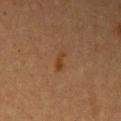The lesion was photographed on a routine skin check and not biopsied; there is no pathology result.
Located on the left upper arm.
The subject is a female in their 60s.
About 2.5 mm across.
A lesion tile, about 15 mm wide, cut from a 3D total-body photograph.